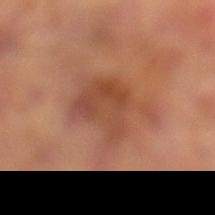Notes:
– follow-up — no biopsy performed (imaged during a skin exam)
– subject — male, aged 63 to 67
– site — the left lower leg
– lesion diameter — ~6 mm (longest diameter)
– automated lesion analysis — an automated nevus-likeness rating near 0 out of 100 and a detector confidence of about 100 out of 100 that the crop contains a lesion
– tile lighting — cross-polarized
– acquisition — 15 mm crop, total-body photography